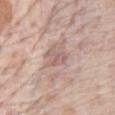{
  "biopsy_status": "not biopsied; imaged during a skin examination",
  "site": "front of the torso",
  "lighting": "white-light",
  "lesion_size": {
    "long_diameter_mm_approx": 2.5
  },
  "patient": {
    "sex": "male",
    "age_approx": "85+"
  },
  "image": {
    "source": "total-body photography crop",
    "field_of_view_mm": 15
  },
  "automated_metrics": {
    "area_mm2_approx": 4.0,
    "eccentricity": 0.6,
    "cielab_L": 59,
    "cielab_a": 18,
    "cielab_b": 21,
    "vs_skin_darker_L": 8.0,
    "border_irregularity_0_10": 4.5,
    "color_variation_0_10": 1.5,
    "peripheral_color_asymmetry": 0.5,
    "nevus_likeness_0_100": 0,
    "lesion_detection_confidence_0_100": 80
  }
}